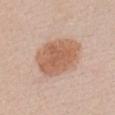| feature | finding |
|---|---|
| workup | total-body-photography surveillance lesion; no biopsy |
| imaging modality | total-body-photography crop, ~15 mm field of view |
| illumination | white-light illumination |
| lesion size | ~6.5 mm (longest diameter) |
| site | the chest |
| patient | female, aged around 40 |